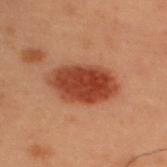<tbp_lesion>
  <automated_metrics>
    <border_irregularity_0_10>1.5</border_irregularity_0_10>
    <nevus_likeness_0_100>100</nevus_likeness_0_100>
    <lesion_detection_confidence_0_100>100</lesion_detection_confidence_0_100>
  </automated_metrics>
  <patient>
    <sex>male</sex>
    <age_approx>55</age_approx>
  </patient>
  <site>upper back</site>
  <image>
    <source>total-body photography crop</source>
    <field_of_view_mm>15</field_of_view_mm>
  </image>
  <lesion_size>
    <long_diameter_mm_approx>7.0</long_diameter_mm_approx>
  </lesion_size>
  <lighting>cross-polarized</lighting>
</tbp_lesion>